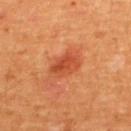Assessment:
Captured during whole-body skin photography for melanoma surveillance; the lesion was not biopsied.
Clinical summary:
This is a cross-polarized tile. About 4 mm across. A 15 mm close-up extracted from a 3D total-body photography capture. A male subject. Located on the upper back.